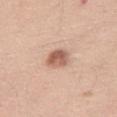{
  "image": {
    "source": "total-body photography crop",
    "field_of_view_mm": 15
  },
  "lesion_size": {
    "long_diameter_mm_approx": 3.0
  },
  "site": "right thigh",
  "lighting": "white-light",
  "patient": {
    "sex": "female",
    "age_approx": 40
  }
}